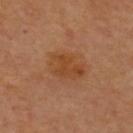Imaged during a routine full-body skin examination; the lesion was not biopsied and no histopathology is available.
Approximately 4.5 mm at its widest.
On the upper back.
A male patient, aged approximately 65.
A 15 mm crop from a total-body photograph taken for skin-cancer surveillance.
Imaged with cross-polarized lighting.